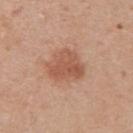From the upper back.
A female patient aged 38 to 42.
Automated image analysis of the tile measured a shape-asymmetry score of about 0.3 (0 = symmetric). The analysis additionally found radial color variation of about 1. And it measured a classifier nevus-likeness of about 55/100.
Cropped from a total-body skin-imaging series; the visible field is about 15 mm.